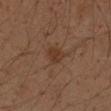biopsy status: total-body-photography surveillance lesion; no biopsy
body site: the left forearm
lesion size: ~2.5 mm (longest diameter)
illumination: cross-polarized
automated lesion analysis: an area of roughly 4 mm²; a mean CIELAB color near L≈31 a*≈17 b*≈25, roughly 6 lightness units darker than nearby skin, and a normalized lesion–skin contrast near 6.5; a color-variation rating of about 1.5/10 and peripheral color asymmetry of about 0.5; a nevus-likeness score of about 20/100 and lesion-presence confidence of about 100/100
acquisition: ~15 mm tile from a whole-body skin photo
patient: male, in their 50s